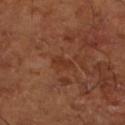biopsy status: catalogued during a skin exam; not biopsied
site: the left lower leg
lesion size: about 2.5 mm
TBP lesion metrics: a lesion color around L≈34 a*≈24 b*≈31 in CIELAB, roughly 6 lightness units darker than nearby skin, and a lesion-to-skin contrast of about 5.5 (normalized; higher = more distinct); a border-irregularity index near 4/10, a within-lesion color-variation index near 0/10, and a peripheral color-asymmetry measure near 0; a classifier nevus-likeness of about 0/100
patient: male, aged 63 to 67
image source: ~15 mm crop, total-body skin-cancer survey